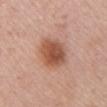<record>
  <patient>
    <sex>female</sex>
    <age_approx>50</age_approx>
  </patient>
  <image>
    <source>total-body photography crop</source>
    <field_of_view_mm>15</field_of_view_mm>
  </image>
  <site>chest</site>
  <lesion_size>
    <long_diameter_mm_approx>4.5</long_diameter_mm_approx>
  </lesion_size>
  <lighting>white-light</lighting>
  <automated_metrics>
    <area_mm2_approx>12.0</area_mm2_approx>
    <vs_skin_darker_L>13.0</vs_skin_darker_L>
    <vs_skin_contrast_norm>9.0</vs_skin_contrast_norm>
    <lesion_detection_confidence_0_100>100</lesion_detection_confidence_0_100>
  </automated_metrics>
</record>